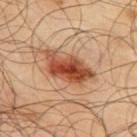Q: Was a biopsy performed?
A: catalogued during a skin exam; not biopsied
Q: What is the imaging modality?
A: ~15 mm tile from a whole-body skin photo
Q: What are the patient's age and sex?
A: male, approximately 65 years of age
Q: What lighting was used for the tile?
A: cross-polarized
Q: Where on the body is the lesion?
A: the upper back